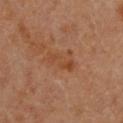- biopsy status: catalogued during a skin exam; not biopsied
- acquisition: ~15 mm crop, total-body skin-cancer survey
- illumination: cross-polarized illumination
- subject: female, in their mid- to late 40s
- lesion size: about 4 mm
- body site: the chest
- automated metrics: a lesion area of about 5 mm² and a symmetry-axis asymmetry near 0.25; internal color variation of about 2 on a 0–10 scale; a nevus-likeness score of about 0/100 and lesion-presence confidence of about 100/100